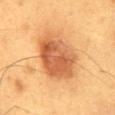{
  "biopsy_status": "not biopsied; imaged during a skin examination",
  "lesion_size": {
    "long_diameter_mm_approx": 6.0
  },
  "site": "back",
  "patient": {
    "sex": "male",
    "age_approx": 60
  },
  "lighting": "cross-polarized",
  "automated_metrics": {
    "area_mm2_approx": 24.0,
    "eccentricity": 0.65,
    "shape_asymmetry": 0.15,
    "color_variation_0_10": 6.5,
    "peripheral_color_asymmetry": 2.0,
    "nevus_likeness_0_100": 100,
    "lesion_detection_confidence_0_100": 100
  },
  "image": {
    "source": "total-body photography crop",
    "field_of_view_mm": 15
  }
}